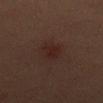Part of a total-body skin-imaging series; this lesion was reviewed on a skin check and was not flagged for biopsy. Longest diameter approximately 3.5 mm. This is a cross-polarized tile. Automated image analysis of the tile measured a footprint of about 7.5 mm², an eccentricity of roughly 0.65, and a symmetry-axis asymmetry near 0.2. And it measured border irregularity of about 2 on a 0–10 scale, a within-lesion color-variation index near 2/10, and peripheral color asymmetry of about 0.5. A roughly 15 mm field-of-view crop from a total-body skin photograph. A female subject roughly 30 years of age. From the abdomen.